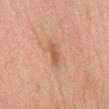{
  "biopsy_status": "not biopsied; imaged during a skin examination",
  "image": {
    "source": "total-body photography crop",
    "field_of_view_mm": 15
  },
  "patient": {
    "sex": "female",
    "age_approx": 65
  },
  "lighting": "white-light",
  "lesion_size": {
    "long_diameter_mm_approx": 2.5
  },
  "site": "mid back"
}